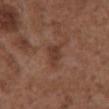notes: total-body-photography surveillance lesion; no biopsy
site: the abdomen
size: ~4 mm (longest diameter)
subject: male, roughly 75 years of age
image source: total-body-photography crop, ~15 mm field of view
TBP lesion metrics: a lesion area of about 6 mm² and an eccentricity of roughly 0.85; an average lesion color of about L≈38 a*≈20 b*≈27 (CIELAB), a lesion–skin lightness drop of about 7, and a normalized lesion–skin contrast near 6.5